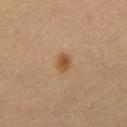follow-up = catalogued during a skin exam; not biopsied
tile lighting = cross-polarized
imaging modality = total-body-photography crop, ~15 mm field of view
subject = female, approximately 50 years of age